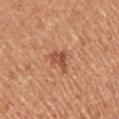follow-up: catalogued during a skin exam; not biopsied.